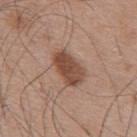Imaged during a routine full-body skin examination; the lesion was not biopsied and no histopathology is available.
On the upper back.
The tile uses white-light illumination.
Longest diameter approximately 4.5 mm.
The patient is a male roughly 55 years of age.
A close-up tile cropped from a whole-body skin photograph, about 15 mm across.